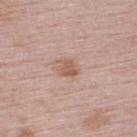This lesion was catalogued during total-body skin photography and was not selected for biopsy. A close-up tile cropped from a whole-body skin photograph, about 15 mm across. From the upper back. A female patient, about 50 years old. The lesion's longest dimension is about 2.5 mm.